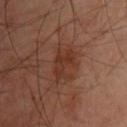{
  "biopsy_status": "not biopsied; imaged during a skin examination",
  "image": {
    "source": "total-body photography crop",
    "field_of_view_mm": 15
  },
  "lesion_size": {
    "long_diameter_mm_approx": 4.0
  },
  "patient": {
    "sex": "male",
    "age_approx": 50
  },
  "site": "chest"
}